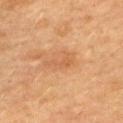Q: Was a biopsy performed?
A: total-body-photography surveillance lesion; no biopsy
Q: What is the anatomic site?
A: the upper back
Q: What did automated image analysis measure?
A: a footprint of about 4.5 mm², an outline eccentricity of about 0.9 (0 = round, 1 = elongated), and two-axis asymmetry of about 0.45; a mean CIELAB color near L≈52 a*≈22 b*≈36 and a normalized border contrast of about 5; an automated nevus-likeness rating near 10 out of 100 and a detector confidence of about 100 out of 100 that the crop contains a lesion
Q: Illumination type?
A: cross-polarized illumination
Q: Who is the patient?
A: female, about 60 years old
Q: What kind of image is this?
A: total-body-photography crop, ~15 mm field of view